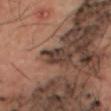Q: Automated lesion metrics?
A: internal color variation of about 2 on a 0–10 scale
Q: Where on the body is the lesion?
A: the right thigh
Q: How large is the lesion?
A: ≈2.5 mm
Q: Illumination type?
A: cross-polarized illumination
Q: Who is the patient?
A: male, aged 63–67
Q: What is the imaging modality?
A: total-body-photography crop, ~15 mm field of view